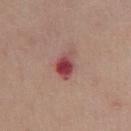follow-up — total-body-photography surveillance lesion; no biopsy | image — total-body-photography crop, ~15 mm field of view | body site — the chest | patient — male, aged around 60.